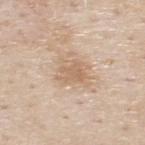biopsy_status: not biopsied; imaged during a skin examination
lesion_size:
  long_diameter_mm_approx: 3.5
automated_metrics:
  border_irregularity_0_10: 3.5
  color_variation_0_10: 2.0
  peripheral_color_asymmetry: 0.5
  lesion_detection_confidence_0_100: 100
image:
  source: total-body photography crop
  field_of_view_mm: 15
lighting: white-light
site: upper back
patient:
  sex: male
  age_approx: 80